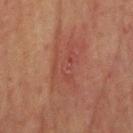{
  "image": {
    "source": "total-body photography crop",
    "field_of_view_mm": 15
  },
  "patient": {
    "sex": "male",
    "age_approx": 70
  },
  "lesion_size": {
    "long_diameter_mm_approx": 6.5
  },
  "site": "head or neck",
  "lighting": "cross-polarized"
}